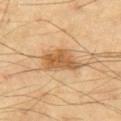Clinical impression:
No biopsy was performed on this lesion — it was imaged during a full skin examination and was not determined to be concerning.
Acquisition and patient details:
A close-up tile cropped from a whole-body skin photograph, about 15 mm across. The lesion is on the left thigh. A male patient, approximately 70 years of age.